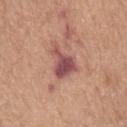Clinical impression:
The lesion was photographed on a routine skin check and not biopsied; there is no pathology result.
Background:
This is a white-light tile. The lesion is on the left forearm. Measured at roughly 4 mm in maximum diameter. A female subject, in their mid- to late 60s. Cropped from a whole-body photographic skin survey; the tile spans about 15 mm.